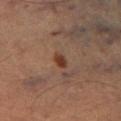| key | value |
|---|---|
| workup | catalogued during a skin exam; not biopsied |
| automated lesion analysis | internal color variation of about 1.5 on a 0–10 scale and a peripheral color-asymmetry measure near 0.5 |
| lighting | cross-polarized |
| patient | male, in their mid-60s |
| image | ~15 mm tile from a whole-body skin photo |
| diameter | ~2 mm (longest diameter) |
| site | the left lower leg |